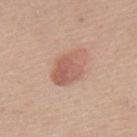Recorded during total-body skin imaging; not selected for excision or biopsy. A roughly 15 mm field-of-view crop from a total-body skin photograph. From the upper back. The tile uses white-light illumination. The recorded lesion diameter is about 5 mm. The total-body-photography lesion software estimated an eccentricity of roughly 0.75 and a symmetry-axis asymmetry near 0.2. It also reported an average lesion color of about L≈58 a*≈23 b*≈27 (CIELAB), a lesion–skin lightness drop of about 10, and a lesion-to-skin contrast of about 6.5 (normalized; higher = more distinct). It also reported a classifier nevus-likeness of about 90/100 and a lesion-detection confidence of about 100/100. The patient is a male aged around 60.